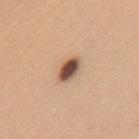workup: total-body-photography surveillance lesion; no biopsy
site: the back
acquisition: ~15 mm crop, total-body skin-cancer survey
size: about 3 mm
lighting: white-light
subject: female, aged 38–42